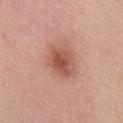workup = total-body-photography surveillance lesion; no biopsy
location = the front of the torso
tile lighting = white-light illumination
subject = female, aged approximately 65
image source = total-body-photography crop, ~15 mm field of view